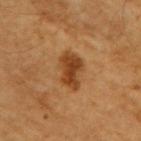biopsy status: no biopsy performed (imaged during a skin exam)
body site: the right upper arm
automated metrics: a footprint of about 9.5 mm², an outline eccentricity of about 0.75 (0 = round, 1 = elongated), and a symmetry-axis asymmetry near 0.25; a mean CIELAB color near L≈39 a*≈22 b*≈36, about 11 CIELAB-L* units darker than the surrounding skin, and a normalized border contrast of about 9; a color-variation rating of about 3.5/10 and radial color variation of about 1
patient: male, roughly 60 years of age
lesion diameter: about 4 mm
imaging modality: total-body-photography crop, ~15 mm field of view
lighting: cross-polarized illumination